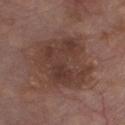Impression:
The lesion was tiled from a total-body skin photograph and was not biopsied.
Image and clinical context:
A male patient, aged around 80. An algorithmic analysis of the crop reported a mean CIELAB color near L≈38 a*≈18 b*≈23, roughly 8 lightness units darker than nearby skin, and a normalized border contrast of about 7. Cropped from a total-body skin-imaging series; the visible field is about 15 mm. Measured at roughly 7 mm in maximum diameter. Located on the chest.